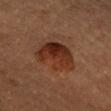Clinical impression: No biopsy was performed on this lesion — it was imaged during a full skin examination and was not determined to be concerning. Acquisition and patient details: Automated tile analysis of the lesion measured a lesion color around L≈24 a*≈20 b*≈24 in CIELAB and roughly 9 lightness units darker than nearby skin. It also reported a border-irregularity index near 3.5/10, internal color variation of about 6.5 on a 0–10 scale, and radial color variation of about 2.5. A 15 mm close-up extracted from a 3D total-body photography capture. A male patient approximately 55 years of age. The tile uses cross-polarized illumination. Located on the upper back.